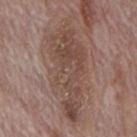- workup — total-body-photography surveillance lesion; no biopsy
- location — the mid back
- lighting — white-light
- automated metrics — a border-irregularity rating of about 6/10, a within-lesion color-variation index near 5/10, and radial color variation of about 2; a classifier nevus-likeness of about 0/100 and a detector confidence of about 50 out of 100 that the crop contains a lesion
- patient — male, aged approximately 70
- acquisition — 15 mm crop, total-body photography
- size — ≈12 mm